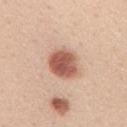Clinical impression:
Imaged during a routine full-body skin examination; the lesion was not biopsied and no histopathology is available.
Image and clinical context:
The subject is a female aged 43–47. This image is a 15 mm lesion crop taken from a total-body photograph. From the mid back. About 4 mm across.